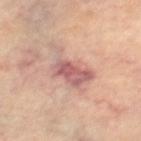<tbp_lesion>
<image>
  <source>total-body photography crop</source>
  <field_of_view_mm>15</field_of_view_mm>
</image>
<lighting>cross-polarized</lighting>
<patient>
  <sex>female</sex>
  <age_approx>65</age_approx>
</patient>
<lesion_size>
  <long_diameter_mm_approx>6.5</long_diameter_mm_approx>
</lesion_size>
<site>right lower leg</site>
</tbp_lesion>